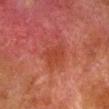Recorded during total-body skin imaging; not selected for excision or biopsy. From the right lower leg. A close-up tile cropped from a whole-body skin photograph, about 15 mm across. This is a cross-polarized tile. The lesion's longest dimension is about 3.5 mm. A male patient aged 78–82.